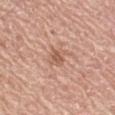Case summary:
– biopsy status: imaged on a skin check; not biopsied
– lesion size: ~2.5 mm (longest diameter)
– anatomic site: the right upper arm
– illumination: white-light
– subject: female, in their mid- to late 60s
– image: ~15 mm crop, total-body skin-cancer survey